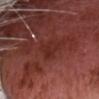On the head or neck. The tile uses white-light illumination. A male subject roughly 75 years of age. Longest diameter approximately 3 mm. A 15 mm crop from a total-body photograph taken for skin-cancer surveillance.